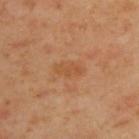Assessment:
No biopsy was performed on this lesion — it was imaged during a full skin examination and was not determined to be concerning.
Clinical summary:
Cropped from a total-body skin-imaging series; the visible field is about 15 mm. The lesion is on the upper back. The lesion's longest dimension is about 3.5 mm. A male subject, aged 43 to 47. Imaged with cross-polarized lighting.